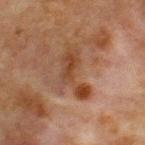Assessment:
The lesion was photographed on a routine skin check and not biopsied; there is no pathology result.
Context:
The lesion is on the chest. The recorded lesion diameter is about 6 mm. Cropped from a whole-body photographic skin survey; the tile spans about 15 mm. The subject is a male aged approximately 65.